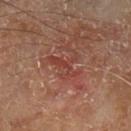workup = total-body-photography surveillance lesion; no biopsy